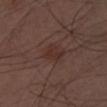Clinical impression: Captured during whole-body skin photography for melanoma surveillance; the lesion was not biopsied. Clinical summary: A male patient aged 48–52. A roughly 15 mm field-of-view crop from a total-body skin photograph.